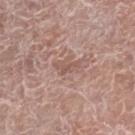Longest diameter approximately 3 mm. Cropped from a total-body skin-imaging series; the visible field is about 15 mm. Imaged with white-light lighting. A male patient, aged 73 to 77. From the right lower leg.